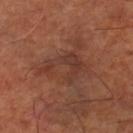The lesion was tiled from a total-body skin photograph and was not biopsied. Longest diameter approximately 5.5 mm. A close-up tile cropped from a whole-body skin photograph, about 15 mm across. Located on the leg. Imaged with cross-polarized lighting. A patient in their mid- to late 60s.